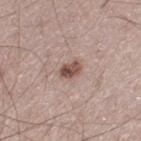Q: Was a biopsy performed?
A: no biopsy performed (imaged during a skin exam)
Q: Who is the patient?
A: male, aged 53–57
Q: How was this image acquired?
A: ~15 mm tile from a whole-body skin photo
Q: What lighting was used for the tile?
A: white-light
Q: Automated lesion metrics?
A: a border-irregularity index near 2/10, internal color variation of about 6.5 on a 0–10 scale, and peripheral color asymmetry of about 3; a classifier nevus-likeness of about 85/100 and a detector confidence of about 100 out of 100 that the crop contains a lesion
Q: What is the anatomic site?
A: the left thigh